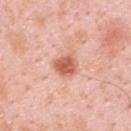Q: Was this lesion biopsied?
A: imaged on a skin check; not biopsied
Q: Lesion location?
A: the upper back
Q: Lesion size?
A: ~3 mm (longest diameter)
Q: How was this image acquired?
A: 15 mm crop, total-body photography
Q: Patient demographics?
A: male, aged 33 to 37
Q: Automated lesion metrics?
A: an automated nevus-likeness rating near 95 out of 100 and lesion-presence confidence of about 100/100
Q: How was the tile lit?
A: white-light illumination